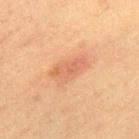Clinical impression: Part of a total-body skin-imaging series; this lesion was reviewed on a skin check and was not flagged for biopsy. Clinical summary: The lesion is on the back. A male subject approximately 60 years of age. A 15 mm close-up tile from a total-body photography series done for melanoma screening. This is a cross-polarized tile.